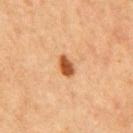The lesion was tiled from a total-body skin photograph and was not biopsied. The patient is a male approximately 65 years of age. A region of skin cropped from a whole-body photographic capture, roughly 15 mm wide. The lesion is on the mid back. Automated image analysis of the tile measured a lesion color around L≈47 a*≈24 b*≈37 in CIELAB, a lesion–skin lightness drop of about 15, and a normalized border contrast of about 11. And it measured a classifier nevus-likeness of about 100/100 and a lesion-detection confidence of about 100/100. This is a cross-polarized tile.